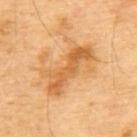Q: Is there a histopathology result?
A: total-body-photography surveillance lesion; no biopsy
Q: How was the tile lit?
A: cross-polarized
Q: What kind of image is this?
A: ~15 mm tile from a whole-body skin photo
Q: What is the anatomic site?
A: the upper back
Q: Patient demographics?
A: male, roughly 70 years of age